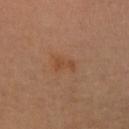Assessment:
Imaged during a routine full-body skin examination; the lesion was not biopsied and no histopathology is available.
Background:
From the arm. A 15 mm crop from a total-body photograph taken for skin-cancer surveillance. A female subject, roughly 50 years of age.